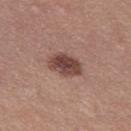  biopsy_status: not biopsied; imaged during a skin examination
  image:
    source: total-body photography crop
    field_of_view_mm: 15
  patient:
    sex: female
    age_approx: 35
  site: left thigh
  lighting: white-light
  lesion_size:
    long_diameter_mm_approx: 4.0